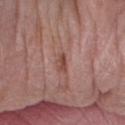Q: Was a biopsy performed?
A: catalogued during a skin exam; not biopsied
Q: What is the imaging modality?
A: 15 mm crop, total-body photography
Q: What is the anatomic site?
A: the right forearm
Q: Automated lesion metrics?
A: a lesion area of about 2.5 mm² and two-axis asymmetry of about 0.25; an automated nevus-likeness rating near 20 out of 100
Q: Who is the patient?
A: male, aged 83 or older
Q: How large is the lesion?
A: ≈2.5 mm